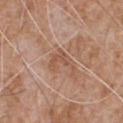Imaged during a routine full-body skin examination; the lesion was not biopsied and no histopathology is available.
A male subject approximately 65 years of age.
The lesion is located on the front of the torso.
A 15 mm crop from a total-body photograph taken for skin-cancer surveillance.